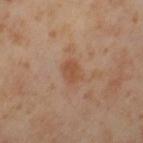workup = imaged on a skin check; not biopsied
anatomic site = the left thigh
diameter = ≈2.5 mm
automated metrics = a border-irregularity index near 2/10, a within-lesion color-variation index near 1.5/10, and radial color variation of about 0.5; lesion-presence confidence of about 100/100
subject = female, in their mid- to late 50s
image = 15 mm crop, total-body photography
tile lighting = cross-polarized illumination